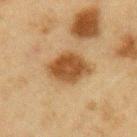Part of a total-body skin-imaging series; this lesion was reviewed on a skin check and was not flagged for biopsy.
Cropped from a whole-body photographic skin survey; the tile spans about 15 mm.
The recorded lesion diameter is about 5.5 mm.
The lesion is located on the right upper arm.
A male subject about 65 years old.
Imaged with cross-polarized lighting.
Automated image analysis of the tile measured an area of roughly 14 mm², an eccentricity of roughly 0.75, and a symmetry-axis asymmetry near 0.15. The analysis additionally found a lesion–skin lightness drop of about 12.